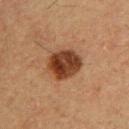Imaged during a routine full-body skin examination; the lesion was not biopsied and no histopathology is available.